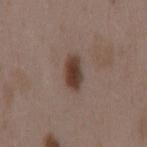Q: Automated lesion metrics?
A: border irregularity of about 2 on a 0–10 scale, internal color variation of about 4 on a 0–10 scale, and peripheral color asymmetry of about 1.5; a classifier nevus-likeness of about 95/100 and lesion-presence confidence of about 100/100
Q: What is the imaging modality?
A: ~15 mm crop, total-body skin-cancer survey
Q: How was the tile lit?
A: white-light illumination
Q: What is the anatomic site?
A: the mid back
Q: How large is the lesion?
A: ~4 mm (longest diameter)
Q: What are the patient's age and sex?
A: female, in their mid- to late 30s